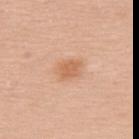The lesion was tiled from a total-body skin photograph and was not biopsied.
This is a white-light tile.
The subject is a female in their mid- to late 50s.
The recorded lesion diameter is about 3 mm.
Automated image analysis of the tile measured a footprint of about 5.5 mm², an eccentricity of roughly 0.7, and two-axis asymmetry of about 0.3. And it measured a within-lesion color-variation index near 2/10.
A lesion tile, about 15 mm wide, cut from a 3D total-body photograph.
On the back.